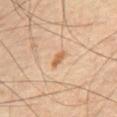biopsy status: total-body-photography surveillance lesion; no biopsy | imaging modality: total-body-photography crop, ~15 mm field of view | body site: the abdomen | automated lesion analysis: a lesion color around L≈53 a*≈18 b*≈32 in CIELAB and roughly 9 lightness units darker than nearby skin; a classifier nevus-likeness of about 60/100 | size: about 2.5 mm | subject: male, roughly 65 years of age.